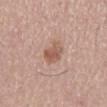Context: The total-body-photography lesion software estimated a mean CIELAB color near L≈56 a*≈21 b*≈27, about 10 CIELAB-L* units darker than the surrounding skin, and a normalized border contrast of about 7. The software also gave a border-irregularity rating of about 2.5/10, internal color variation of about 3 on a 0–10 scale, and peripheral color asymmetry of about 1. A male patient, aged 58 to 62. The lesion is located on the back. This image is a 15 mm lesion crop taken from a total-body photograph. Longest diameter approximately 3.5 mm.